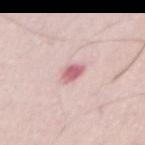– notes — catalogued during a skin exam; not biopsied
– lighting — white-light illumination
– imaging modality — ~15 mm tile from a whole-body skin photo
– size — ≈2.5 mm
– body site — the abdomen
– patient — female, about 45 years old